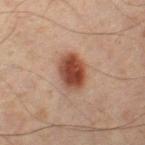The lesion was photographed on a routine skin check and not biopsied; there is no pathology result.
A roughly 15 mm field-of-view crop from a total-body skin photograph.
This is a cross-polarized tile.
The lesion is located on the right thigh.
A male subject aged around 65.
The lesion's longest dimension is about 4 mm.